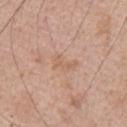Imaged during a routine full-body skin examination; the lesion was not biopsied and no histopathology is available.
The lesion is on the arm.
A 15 mm close-up extracted from a 3D total-body photography capture.
About 3 mm across.
Imaged with white-light lighting.
A male patient aged approximately 65.